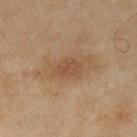Measured at roughly 3.5 mm in maximum diameter. The subject is a female in their 60s. On the left lower leg. This is a cross-polarized tile. A 15 mm close-up extracted from a 3D total-body photography capture. An algorithmic analysis of the crop reported a footprint of about 5.5 mm², an outline eccentricity of about 0.8 (0 = round, 1 = elongated), and a shape-asymmetry score of about 0.25 (0 = symmetric). It also reported a lesion–skin lightness drop of about 7 and a lesion-to-skin contrast of about 5.5 (normalized; higher = more distinct).